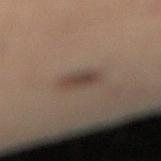Findings:
* workup · imaged on a skin check; not biopsied
* location · the right lower leg
* imaging modality · total-body-photography crop, ~15 mm field of view
* subject · male, aged around 55